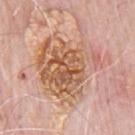No biopsy was performed on this lesion — it was imaged during a full skin examination and was not determined to be concerning.
The patient is a male aged 78–82.
This is a white-light tile.
Longest diameter approximately 8 mm.
A 15 mm close-up tile from a total-body photography series done for melanoma screening.
The lesion is located on the chest.